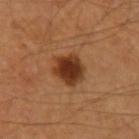workup: no biopsy performed (imaged during a skin exam)
tile lighting: cross-polarized illumination
patient: male, approximately 65 years of age
anatomic site: the left upper arm
image source: 15 mm crop, total-body photography
automated metrics: a border-irregularity rating of about 2/10 and internal color variation of about 4.5 on a 0–10 scale; a nevus-likeness score of about 100/100 and a detector confidence of about 100 out of 100 that the crop contains a lesion
diameter: ≈4 mm